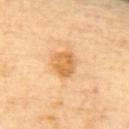Assessment:
Captured during whole-body skin photography for melanoma surveillance; the lesion was not biopsied.
Acquisition and patient details:
A 15 mm crop from a total-body photograph taken for skin-cancer surveillance. Imaged with cross-polarized lighting. About 3 mm across. A female subject in their mid- to late 60s. An algorithmic analysis of the crop reported a footprint of about 7.5 mm² and a shape eccentricity near 0.35. The analysis additionally found an average lesion color of about L≈69 a*≈23 b*≈46 (CIELAB), a lesion–skin lightness drop of about 11, and a normalized border contrast of about 7.5. On the upper back.